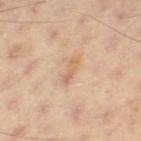| feature | finding |
|---|---|
| imaging modality | ~15 mm crop, total-body skin-cancer survey |
| subject | male, aged 53–57 |
| site | the right thigh |
| tile lighting | cross-polarized |